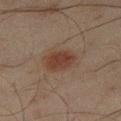Q: Is there a histopathology result?
A: catalogued during a skin exam; not biopsied
Q: What lighting was used for the tile?
A: cross-polarized
Q: What is the imaging modality?
A: total-body-photography crop, ~15 mm field of view
Q: Who is the patient?
A: male, aged approximately 45
Q: What is the anatomic site?
A: the right thigh
Q: Lesion size?
A: about 3.5 mm
Q: Automated lesion metrics?
A: an area of roughly 8.5 mm² and a shape-asymmetry score of about 0.15 (0 = symmetric); an average lesion color of about L≈31 a*≈16 b*≈21 (CIELAB); a border-irregularity index near 1.5/10, a color-variation rating of about 2/10, and radial color variation of about 0.5; a nevus-likeness score of about 100/100